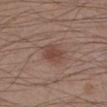follow-up: catalogued during a skin exam; not biopsied
site: the left lower leg
acquisition: 15 mm crop, total-body photography
subject: male, aged approximately 30